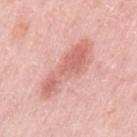– workup · catalogued during a skin exam; not biopsied
– size · about 8 mm
– image source · ~15 mm tile from a whole-body skin photo
– patient · female, roughly 65 years of age
– tile lighting · white-light illumination
– location · the left upper arm